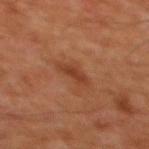This lesion was catalogued during total-body skin photography and was not selected for biopsy.
About 3 mm across.
A male patient, aged around 60.
This image is a 15 mm lesion crop taken from a total-body photograph.
On the chest.